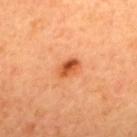Automated image analysis of the tile measured a lesion color around L≈55 a*≈34 b*≈45 in CIELAB, roughly 14 lightness units darker than nearby skin, and a normalized lesion–skin contrast near 9.
A female patient, roughly 45 years of age.
Cropped from a whole-body photographic skin survey; the tile spans about 15 mm.
On the upper back.
Approximately 2.5 mm at its widest.